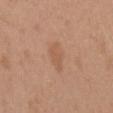Assessment:
Recorded during total-body skin imaging; not selected for excision or biopsy.
Background:
From the mid back. The lesion's longest dimension is about 3.5 mm. Automated image analysis of the tile measured a footprint of about 4 mm², an outline eccentricity of about 0.9 (0 = round, 1 = elongated), and a shape-asymmetry score of about 0.35 (0 = symmetric). It also reported a lesion color around L≈56 a*≈21 b*≈33 in CIELAB and a lesion–skin lightness drop of about 6. The tile uses white-light illumination. A female patient, aged around 40. Cropped from a total-body skin-imaging series; the visible field is about 15 mm.